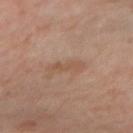biopsy status: catalogued during a skin exam; not biopsied
lesion size: ~4 mm (longest diameter)
anatomic site: the arm
imaging modality: 15 mm crop, total-body photography
illumination: cross-polarized illumination
subject: female, in their 40s
TBP lesion metrics: border irregularity of about 4.5 on a 0–10 scale, internal color variation of about 0 on a 0–10 scale, and peripheral color asymmetry of about 0; a nevus-likeness score of about 0/100 and lesion-presence confidence of about 100/100